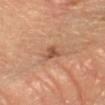No biopsy was performed on this lesion — it was imaged during a full skin examination and was not determined to be concerning.
The lesion is on the left forearm.
A roughly 15 mm field-of-view crop from a total-body skin photograph.
A female patient in their 60s.